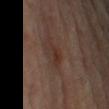Assessment: Captured during whole-body skin photography for melanoma surveillance; the lesion was not biopsied. Clinical summary: Captured under cross-polarized illumination. Longest diameter approximately 3 mm. Cropped from a whole-body photographic skin survey; the tile spans about 15 mm. A male patient aged 63 to 67. The lesion is located on the left upper arm. The lesion-visualizer software estimated a normalized border contrast of about 6.5. The software also gave an automated nevus-likeness rating near 5 out of 100.